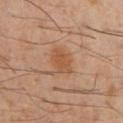Imaged during a routine full-body skin examination; the lesion was not biopsied and no histopathology is available. The total-body-photography lesion software estimated a classifier nevus-likeness of about 85/100 and a detector confidence of about 100 out of 100 that the crop contains a lesion. Approximately 3.5 mm at its widest. Captured under cross-polarized illumination. On the chest. A male subject aged 58 to 62. A roughly 15 mm field-of-view crop from a total-body skin photograph.